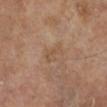– location — the left lower leg
– automated lesion analysis — an area of roughly 3.5 mm², an eccentricity of roughly 0.8, and a shape-asymmetry score of about 0.45 (0 = symmetric); a mean CIELAB color near L≈51 a*≈17 b*≈31 and a lesion–skin lightness drop of about 5; a within-lesion color-variation index near 1.5/10 and a peripheral color-asymmetry measure near 0.5; a classifier nevus-likeness of about 0/100 and a detector confidence of about 100 out of 100 that the crop contains a lesion
– patient — female, in their mid- to late 70s
– diameter — ~3 mm (longest diameter)
– illumination — cross-polarized illumination
– imaging modality — 15 mm crop, total-body photography